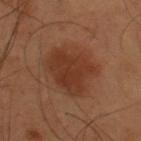Imaged during a routine full-body skin examination; the lesion was not biopsied and no histopathology is available.
This image is a 15 mm lesion crop taken from a total-body photograph.
The lesion-visualizer software estimated a lesion area of about 23 mm² and a symmetry-axis asymmetry near 0.25. The software also gave a border-irregularity rating of about 2.5/10, a within-lesion color-variation index near 2.5/10, and a peripheral color-asymmetry measure near 1. And it measured an automated nevus-likeness rating near 85 out of 100 and a lesion-detection confidence of about 100/100.
The patient is a male approximately 55 years of age.
The lesion is located on the upper back.
Imaged with cross-polarized lighting.
The lesion's longest dimension is about 6 mm.